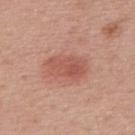{"biopsy_status": "not biopsied; imaged during a skin examination", "automated_metrics": {"nevus_likeness_0_100": 95, "lesion_detection_confidence_0_100": 100}, "lesion_size": {"long_diameter_mm_approx": 5.0}, "patient": {"sex": "male", "age_approx": 65}, "image": {"source": "total-body photography crop", "field_of_view_mm": 15}, "site": "upper back"}